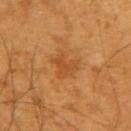No biopsy was performed on this lesion — it was imaged during a full skin examination and was not determined to be concerning.
A 15 mm close-up extracted from a 3D total-body photography capture.
Measured at roughly 3.5 mm in maximum diameter.
Captured under cross-polarized illumination.
The lesion is on the arm.
A male patient in their 60s.